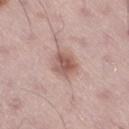Q: What are the patient's age and sex?
A: male, aged 53 to 57
Q: What is the anatomic site?
A: the right thigh
Q: What is the imaging modality?
A: ~15 mm tile from a whole-body skin photo
Q: Lesion size?
A: ≈3 mm